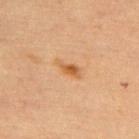Q: Was this lesion biopsied?
A: no biopsy performed (imaged during a skin exam)
Q: What is the anatomic site?
A: the upper back
Q: How was this image acquired?
A: ~15 mm crop, total-body skin-cancer survey
Q: What is the lesion's diameter?
A: ~3 mm (longest diameter)
Q: Patient demographics?
A: female, roughly 65 years of age
Q: What lighting was used for the tile?
A: cross-polarized